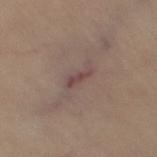Imaged during a routine full-body skin examination; the lesion was not biopsied and no histopathology is available.